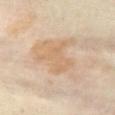No biopsy was performed on this lesion — it was imaged during a full skin examination and was not determined to be concerning. Longest diameter approximately 4.5 mm. A roughly 15 mm field-of-view crop from a total-body skin photograph. Imaged with cross-polarized lighting. The total-body-photography lesion software estimated an average lesion color of about L≈67 a*≈17 b*≈35 (CIELAB), about 7 CIELAB-L* units darker than the surrounding skin, and a normalized lesion–skin contrast near 5. And it measured a border-irregularity rating of about 7.5/10, a within-lesion color-variation index near 2/10, and radial color variation of about 0.5. And it measured a nevus-likeness score of about 0/100. The lesion is located on the abdomen. A female patient in their mid-50s.